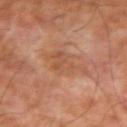Captured under cross-polarized illumination.
A male patient aged 68 to 72.
A region of skin cropped from a whole-body photographic capture, roughly 15 mm wide.
From the right upper arm.
About 5 mm across.
An algorithmic analysis of the crop reported a lesion–skin lightness drop of about 6 and a normalized border contrast of about 5.